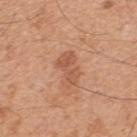Captured during whole-body skin photography for melanoma surveillance; the lesion was not biopsied.